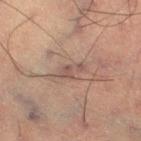Clinical impression:
Captured during whole-body skin photography for melanoma surveillance; the lesion was not biopsied.
Context:
The tile uses cross-polarized illumination. Approximately 3 mm at its widest. Cropped from a whole-body photographic skin survey; the tile spans about 15 mm. An algorithmic analysis of the crop reported a lesion color around L≈45 a*≈16 b*≈20 in CIELAB and a normalized border contrast of about 6. It also reported a border-irregularity rating of about 4.5/10 and internal color variation of about 2 on a 0–10 scale. The analysis additionally found a detector confidence of about 60 out of 100 that the crop contains a lesion. A male patient, roughly 60 years of age.